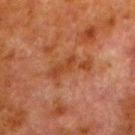Background: Captured under cross-polarized illumination. A 15 mm close-up extracted from a 3D total-body photography capture. On the left lower leg. Automated image analysis of the tile measured an average lesion color of about L≈35 a*≈22 b*≈31 (CIELAB), a lesion–skin lightness drop of about 6, and a normalized lesion–skin contrast near 7. The analysis additionally found a color-variation rating of about 2/10 and peripheral color asymmetry of about 0.5. A male subject, about 80 years old. Approximately 5 mm at its widest.